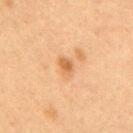Recorded during total-body skin imaging; not selected for excision or biopsy.
About 2.5 mm across.
A female patient, aged 38 to 42.
A close-up tile cropped from a whole-body skin photograph, about 15 mm across.
Imaged with cross-polarized lighting.
Located on the upper back.